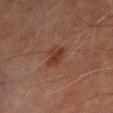Background:
The patient is a male roughly 70 years of age. The lesion's longest dimension is about 3 mm. The lesion is on the leg. A 15 mm close-up tile from a total-body photography series done for melanoma screening.